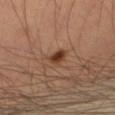Impression: Recorded during total-body skin imaging; not selected for excision or biopsy. Acquisition and patient details: Located on the right thigh. A male patient, aged 53 to 57. Cropped from a total-body skin-imaging series; the visible field is about 15 mm. This is a cross-polarized tile.